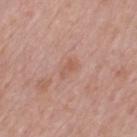anatomic site: the mid back
subject: male, approximately 55 years of age
illumination: white-light illumination
acquisition: total-body-photography crop, ~15 mm field of view